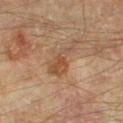follow-up = catalogued during a skin exam; not biopsied | subject = in their mid- to late 50s | body site = the left lower leg | acquisition = total-body-photography crop, ~15 mm field of view.